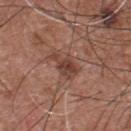Clinical impression:
Captured during whole-body skin photography for melanoma surveillance; the lesion was not biopsied.
Image and clinical context:
An algorithmic analysis of the crop reported an average lesion color of about L≈42 a*≈22 b*≈26 (CIELAB) and a lesion-to-skin contrast of about 7.5 (normalized; higher = more distinct). It also reported a border-irregularity index near 5/10, a color-variation rating of about 4.5/10, and a peripheral color-asymmetry measure near 1.5. The analysis additionally found a nevus-likeness score of about 25/100 and lesion-presence confidence of about 100/100. A male subject approximately 55 years of age. From the chest. A lesion tile, about 15 mm wide, cut from a 3D total-body photograph. Approximately 3.5 mm at its widest.